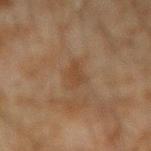Impression:
The lesion was tiled from a total-body skin photograph and was not biopsied.
Context:
Automated image analysis of the tile measured an area of roughly 5.5 mm², an outline eccentricity of about 0.8 (0 = round, 1 = elongated), and a symmetry-axis asymmetry near 0.3. The software also gave a lesion color around L≈36 a*≈14 b*≈27 in CIELAB, a lesion–skin lightness drop of about 5, and a normalized border contrast of about 5. It also reported internal color variation of about 1.5 on a 0–10 scale and radial color variation of about 0.5. It also reported an automated nevus-likeness rating near 0 out of 100. Approximately 3.5 mm at its widest. Imaged with cross-polarized lighting. A male patient, aged approximately 45. This image is a 15 mm lesion crop taken from a total-body photograph. The lesion is on the left forearm.